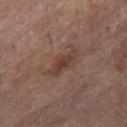Clinical impression:
The lesion was tiled from a total-body skin photograph and was not biopsied.
Context:
The total-body-photography lesion software estimated a lesion area of about 6.5 mm², a shape eccentricity near 0.85, and a shape-asymmetry score of about 0.35 (0 = symmetric). The software also gave a mean CIELAB color near L≈35 a*≈17 b*≈22, a lesion–skin lightness drop of about 6, and a normalized border contrast of about 6.5. And it measured a border-irregularity index near 4/10, a within-lesion color-variation index near 2.5/10, and peripheral color asymmetry of about 0.5. The analysis additionally found an automated nevus-likeness rating near 10 out of 100. The lesion is located on the left leg. This is a cross-polarized tile. Cropped from a total-body skin-imaging series; the visible field is about 15 mm. The subject is a female about 80 years old.